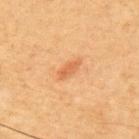image-analysis metrics: a lesion color around L≈57 a*≈25 b*≈39 in CIELAB, a lesion–skin lightness drop of about 9, and a lesion-to-skin contrast of about 6 (normalized; higher = more distinct); a nevus-likeness score of about 75/100
lesion diameter: ~3 mm (longest diameter)
anatomic site: the front of the torso
image source: 15 mm crop, total-body photography
subject: male, about 60 years old
tile lighting: cross-polarized